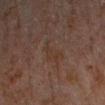Clinical impression: This lesion was catalogued during total-body skin photography and was not selected for biopsy. Background: On the left forearm. The subject is a male aged around 30. Cropped from a whole-body photographic skin survey; the tile spans about 15 mm. The lesion-visualizer software estimated a mean CIELAB color near L≈27 a*≈13 b*≈21. And it measured a classifier nevus-likeness of about 0/100.